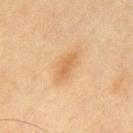Assessment: Part of a total-body skin-imaging series; this lesion was reviewed on a skin check and was not flagged for biopsy. Image and clinical context: From the mid back. A lesion tile, about 15 mm wide, cut from a 3D total-body photograph. Automated image analysis of the tile measured border irregularity of about 3 on a 0–10 scale, a color-variation rating of about 2/10, and peripheral color asymmetry of about 0.5. The analysis additionally found a nevus-likeness score of about 75/100 and lesion-presence confidence of about 100/100. A male subject approximately 45 years of age.